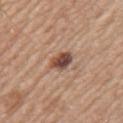notes: total-body-photography surveillance lesion; no biopsy
subject: male, aged around 65
site: the left upper arm
tile lighting: white-light illumination
lesion diameter: about 3 mm
imaging modality: total-body-photography crop, ~15 mm field of view